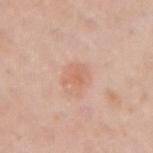Assessment:
Part of a total-body skin-imaging series; this lesion was reviewed on a skin check and was not flagged for biopsy.
Clinical summary:
The patient is a female aged 43–47. The recorded lesion diameter is about 3 mm. The lesion is located on the right upper arm. This is a white-light tile. A 15 mm close-up extracted from a 3D total-body photography capture.